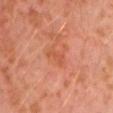- notes — total-body-photography surveillance lesion; no biopsy
- subject — male, roughly 30 years of age
- location — the left upper arm
- image source — total-body-photography crop, ~15 mm field of view
- illumination — cross-polarized